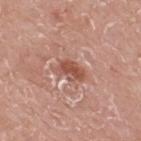<record>
<image>
  <source>total-body photography crop</source>
  <field_of_view_mm>15</field_of_view_mm>
</image>
<site>upper back</site>
<lighting>white-light</lighting>
<patient>
  <sex>male</sex>
  <age_approx>75</age_approx>
</patient>
</record>